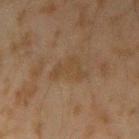Case summary:
– follow-up · catalogued during a skin exam; not biopsied
– imaging modality · ~15 mm crop, total-body skin-cancer survey
– subject · male, aged approximately 45
– body site · the left forearm
– diameter · about 4.5 mm
– lighting · cross-polarized